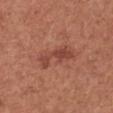follow-up=total-body-photography surveillance lesion; no biopsy
patient=female, aged 48–52
site=the chest
image=total-body-photography crop, ~15 mm field of view